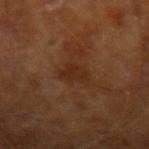  biopsy_status: not biopsied; imaged during a skin examination
  site: left forearm
  patient:
    sex: male
    age_approx: 65
  image:
    source: total-body photography crop
    field_of_view_mm: 15
  lesion_size:
    long_diameter_mm_approx: 3.5
  lighting: cross-polarized